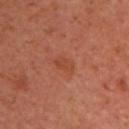Findings:
• notes: no biopsy performed (imaged during a skin exam)
• image source: ~15 mm tile from a whole-body skin photo
• image-analysis metrics: a border-irregularity rating of about 2.5/10, a color-variation rating of about 1.5/10, and radial color variation of about 0.5
• patient: female, aged 38–42
• location: the chest
• illumination: cross-polarized illumination